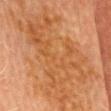Impression: This lesion was catalogued during total-body skin photography and was not selected for biopsy. Image and clinical context: The recorded lesion diameter is about 14.5 mm. The tile uses cross-polarized illumination. A roughly 15 mm field-of-view crop from a total-body skin photograph. The lesion is on the head or neck. A male subject aged 68 to 72.